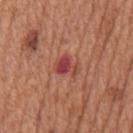Q: Is there a histopathology result?
A: catalogued during a skin exam; not biopsied
Q: How was this image acquired?
A: ~15 mm crop, total-body skin-cancer survey
Q: What are the patient's age and sex?
A: male, aged around 65
Q: What is the lesion's diameter?
A: about 3 mm
Q: What lighting was used for the tile?
A: white-light illumination
Q: Where on the body is the lesion?
A: the mid back